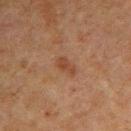Q: Was a biopsy performed?
A: no biopsy performed (imaged during a skin exam)
Q: What is the lesion's diameter?
A: ≈3 mm
Q: Automated lesion metrics?
A: an eccentricity of roughly 0.9 and two-axis asymmetry of about 0.3
Q: Lesion location?
A: the left arm
Q: Who is the patient?
A: male, about 60 years old
Q: How was the tile lit?
A: cross-polarized illumination
Q: How was this image acquired?
A: total-body-photography crop, ~15 mm field of view